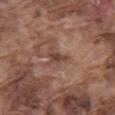Assessment:
Imaged during a routine full-body skin examination; the lesion was not biopsied and no histopathology is available.
Background:
From the mid back. The tile uses white-light illumination. Longest diameter approximately 3 mm. A 15 mm crop from a total-body photograph taken for skin-cancer surveillance. A male subject approximately 75 years of age. The total-body-photography lesion software estimated a classifier nevus-likeness of about 0/100 and a detector confidence of about 90 out of 100 that the crop contains a lesion.